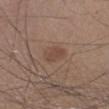image:
  source: total-body photography crop
  field_of_view_mm: 15
patient:
  sex: male
  age_approx: 45
site: left lower leg
lighting: white-light
automated_metrics:
  cielab_L: 46
  cielab_a: 17
  cielab_b: 26
  vs_skin_darker_L: 7.0
  border_irregularity_0_10: 2.0
  color_variation_0_10: 2.0
  peripheral_color_asymmetry: 0.5
  nevus_likeness_0_100: 30
  lesion_detection_confidence_0_100: 100
lesion_size:
  long_diameter_mm_approx: 3.0